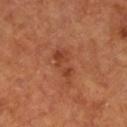Q: Is there a histopathology result?
A: imaged on a skin check; not biopsied
Q: What lighting was used for the tile?
A: cross-polarized
Q: Where on the body is the lesion?
A: the leg
Q: What is the imaging modality?
A: ~15 mm tile from a whole-body skin photo
Q: Patient demographics?
A: male, aged around 65
Q: Lesion size?
A: ≈4 mm